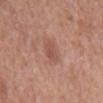| feature | finding |
|---|---|
| follow-up | catalogued during a skin exam; not biopsied |
| lighting | white-light illumination |
| patient | male, in their mid-60s |
| location | the mid back |
| size | ~2.5 mm (longest diameter) |
| acquisition | ~15 mm crop, total-body skin-cancer survey |